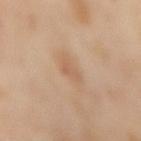Clinical impression: Part of a total-body skin-imaging series; this lesion was reviewed on a skin check and was not flagged for biopsy. Clinical summary: A female patient, aged 53–57. On the mid back. Imaged with cross-polarized lighting. An algorithmic analysis of the crop reported a shape-asymmetry score of about 0.35 (0 = symmetric). The analysis additionally found an average lesion color of about L≈60 a*≈19 b*≈33 (CIELAB), a lesion–skin lightness drop of about 7, and a normalized lesion–skin contrast near 4.5. The analysis additionally found a border-irregularity index near 3.5/10, a color-variation rating of about 2/10, and radial color variation of about 0.5. A 15 mm close-up tile from a total-body photography series done for melanoma screening. Longest diameter approximately 2.5 mm.